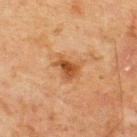{
  "lesion_size": {
    "long_diameter_mm_approx": 3.5
  },
  "image": {
    "source": "total-body photography crop",
    "field_of_view_mm": 15
  },
  "patient": {
    "sex": "male",
    "age_approx": 70
  },
  "lighting": "cross-polarized",
  "automated_metrics": {
    "area_mm2_approx": 7.5,
    "eccentricity": 0.7,
    "shape_asymmetry": 0.35,
    "vs_skin_contrast_norm": 8.0,
    "color_variation_0_10": 4.0,
    "peripheral_color_asymmetry": 1.0
  },
  "site": "upper back"
}